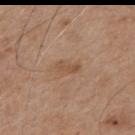Cropped from a whole-body photographic skin survey; the tile spans about 15 mm.
Measured at roughly 3 mm in maximum diameter.
A male subject, roughly 65 years of age.
The lesion is located on the back.